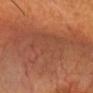{
  "biopsy_status": "not biopsied; imaged during a skin examination",
  "lighting": "cross-polarized",
  "image": {
    "source": "total-body photography crop",
    "field_of_view_mm": 15
  },
  "patient": {
    "sex": "male",
    "age_approx": 65
  },
  "site": "head or neck"
}